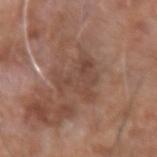biopsy status — imaged on a skin check; not biopsied
acquisition — 15 mm crop, total-body photography
patient — male, aged approximately 75
size — ≈5 mm
illumination — white-light
site — the right forearm